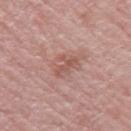Q: Is there a histopathology result?
A: no biopsy performed (imaged during a skin exam)
Q: How was this image acquired?
A: 15 mm crop, total-body photography
Q: What is the anatomic site?
A: the right upper arm
Q: How large is the lesion?
A: about 3 mm
Q: Who is the patient?
A: female, in their mid- to late 60s
Q: What lighting was used for the tile?
A: white-light